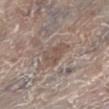Case summary:
- body site: the left lower leg
- size: about 3.5 mm
- image source: ~15 mm crop, total-body skin-cancer survey
- patient: female, roughly 85 years of age or older
- tile lighting: white-light